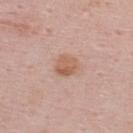<case>
<biopsy_status>not biopsied; imaged during a skin examination</biopsy_status>
<automated_metrics>
  <area_mm2_approx>6.0</area_mm2_approx>
  <eccentricity>0.45</eccentricity>
  <shape_asymmetry>0.25</shape_asymmetry>
  <cielab_L>59</cielab_L>
  <cielab_a>21</cielab_a>
  <cielab_b>30</cielab_b>
  <vs_skin_darker_L>9.0</vs_skin_darker_L>
  <vs_skin_contrast_norm>7.0</vs_skin_contrast_norm>
  <border_irregularity_0_10>2.5</border_irregularity_0_10>
  <color_variation_0_10>4.0</color_variation_0_10>
  <peripheral_color_asymmetry>1.5</peripheral_color_asymmetry>
</automated_metrics>
<site>back</site>
<patient>
  <sex>male</sex>
  <age_approx>25</age_approx>
</patient>
<lighting>white-light</lighting>
<lesion_size>
  <long_diameter_mm_approx>3.0</long_diameter_mm_approx>
</lesion_size>
<image>
  <source>total-body photography crop</source>
  <field_of_view_mm>15</field_of_view_mm>
</image>
</case>